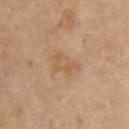Q: Was a biopsy performed?
A: total-body-photography surveillance lesion; no biopsy
Q: What kind of image is this?
A: total-body-photography crop, ~15 mm field of view
Q: Lesion location?
A: the left upper arm
Q: Patient demographics?
A: female, in their mid-60s